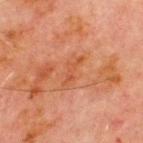Imaged during a routine full-body skin examination; the lesion was not biopsied and no histopathology is available.
The subject is a male aged 68–72.
From the chest.
Captured under cross-polarized illumination.
Automated image analysis of the tile measured a shape eccentricity near 0.95 and two-axis asymmetry of about 0.55. The analysis additionally found an average lesion color of about L≈44 a*≈25 b*≈33 (CIELAB), about 6 CIELAB-L* units darker than the surrounding skin, and a lesion-to-skin contrast of about 5.5 (normalized; higher = more distinct). The analysis additionally found a border-irregularity index near 6.5/10 and radial color variation of about 0.
A close-up tile cropped from a whole-body skin photograph, about 15 mm across.